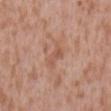Part of a total-body skin-imaging series; this lesion was reviewed on a skin check and was not flagged for biopsy. The lesion-visualizer software estimated a lesion area of about 4.5 mm² and two-axis asymmetry of about 0.4. And it measured a border-irregularity rating of about 4.5/10, internal color variation of about 2.5 on a 0–10 scale, and radial color variation of about 1. This is a white-light tile. A male subject in their mid- to late 40s. About 3.5 mm across. A region of skin cropped from a whole-body photographic capture, roughly 15 mm wide. The lesion is located on the mid back.